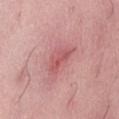Clinical impression: The lesion was tiled from a total-body skin photograph and was not biopsied.